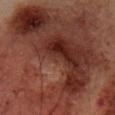Q: Was this lesion biopsied?
A: no biopsy performed (imaged during a skin exam)
Q: How was this image acquired?
A: 15 mm crop, total-body photography
Q: Illumination type?
A: cross-polarized
Q: What are the patient's age and sex?
A: male, about 55 years old
Q: What did automated image analysis measure?
A: a lesion area of about 130 mm², an outline eccentricity of about 0.85 (0 = round, 1 = elongated), and a shape-asymmetry score of about 0.4 (0 = symmetric); a nevus-likeness score of about 0/100 and a lesion-detection confidence of about 0/100
Q: Lesion size?
A: ~19.5 mm (longest diameter)
Q: What is the anatomic site?
A: the front of the torso